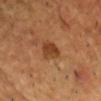Imaged during a routine full-body skin examination; the lesion was not biopsied and no histopathology is available. The lesion is located on the front of the torso. Automated image analysis of the tile measured a lesion color around L≈42 a*≈23 b*≈36 in CIELAB, roughly 10 lightness units darker than nearby skin, and a lesion-to-skin contrast of about 8 (normalized; higher = more distinct). The analysis additionally found a classifier nevus-likeness of about 75/100 and lesion-presence confidence of about 100/100. The recorded lesion diameter is about 3.5 mm. A male patient in their mid-40s. A lesion tile, about 15 mm wide, cut from a 3D total-body photograph.